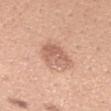Assessment:
Captured during whole-body skin photography for melanoma surveillance; the lesion was not biopsied.
Background:
About 3.5 mm across. A female subject, about 30 years old. The tile uses white-light illumination. Cropped from a total-body skin-imaging series; the visible field is about 15 mm. The lesion is on the right forearm.